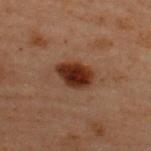Impression:
The lesion was photographed on a routine skin check and not biopsied; there is no pathology result.
Background:
This image is a 15 mm lesion crop taken from a total-body photograph. The lesion is located on the upper back. Automated tile analysis of the lesion measured a nevus-likeness score of about 100/100 and lesion-presence confidence of about 100/100. Measured at roughly 4 mm in maximum diameter. A male patient aged around 60. Imaged with cross-polarized lighting.